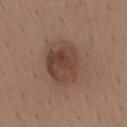Notes:
- automated lesion analysis — an area of roughly 20 mm² and a shape eccentricity near 0.6; border irregularity of about 1 on a 0–10 scale; lesion-presence confidence of about 100/100
- acquisition — ~15 mm crop, total-body skin-cancer survey
- body site — the mid back
- patient — male, aged around 40
- tile lighting — white-light
- lesion size — about 5.5 mm